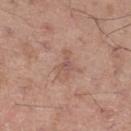Background: A 15 mm crop from a total-body photograph taken for skin-cancer surveillance. A male patient aged approximately 55. Approximately 3 mm at its widest. An algorithmic analysis of the crop reported border irregularity of about 5.5 on a 0–10 scale and a within-lesion color-variation index near 2.5/10. The analysis additionally found a classifier nevus-likeness of about 0/100. Located on the leg.